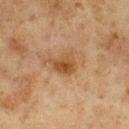Captured during whole-body skin photography for melanoma surveillance; the lesion was not biopsied.
The lesion is located on the chest.
A male patient aged 73 to 77.
Cropped from a total-body skin-imaging series; the visible field is about 15 mm.